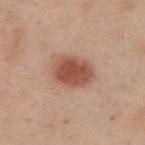No biopsy was performed on this lesion — it was imaged during a full skin examination and was not determined to be concerning.
The lesion is on the upper back.
Captured under white-light illumination.
A male patient aged 38–42.
Longest diameter approximately 4.5 mm.
Automated image analysis of the tile measured an automated nevus-likeness rating near 100 out of 100.
Cropped from a whole-body photographic skin survey; the tile spans about 15 mm.